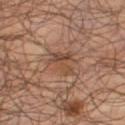Captured during whole-body skin photography for melanoma surveillance; the lesion was not biopsied.
A male subject, about 65 years old.
A lesion tile, about 15 mm wide, cut from a 3D total-body photograph.
On the right thigh.